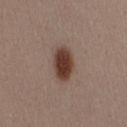Part of a total-body skin-imaging series; this lesion was reviewed on a skin check and was not flagged for biopsy. This image is a 15 mm lesion crop taken from a total-body photograph. About 4 mm across. The total-body-photography lesion software estimated a mean CIELAB color near L≈39 a*≈18 b*≈25 and a lesion–skin lightness drop of about 15. The tile uses white-light illumination. A female subject, in their 30s. Located on the right thigh.